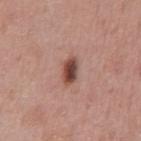Part of a total-body skin-imaging series; this lesion was reviewed on a skin check and was not flagged for biopsy. The lesion is on the abdomen. The tile uses white-light illumination. This image is a 15 mm lesion crop taken from a total-body photograph. Longest diameter approximately 3.5 mm. A male subject, aged 53 to 57.